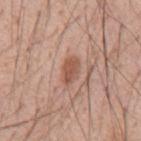Clinical impression: No biopsy was performed on this lesion — it was imaged during a full skin examination and was not determined to be concerning. Image and clinical context: A close-up tile cropped from a whole-body skin photograph, about 15 mm across. Located on the back. Measured at roughly 3 mm in maximum diameter. Imaged with white-light lighting. Automated tile analysis of the lesion measured a lesion area of about 5 mm², a shape eccentricity near 0.8, and two-axis asymmetry of about 0.15. The software also gave a lesion color around L≈54 a*≈22 b*≈29 in CIELAB, a lesion–skin lightness drop of about 10, and a normalized border contrast of about 7.5. The software also gave a nevus-likeness score of about 85/100 and lesion-presence confidence of about 100/100. The patient is a male approximately 55 years of age.